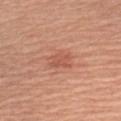Cropped from a whole-body photographic skin survey; the tile spans about 15 mm. The lesion-visualizer software estimated a border-irregularity index near 3/10, a color-variation rating of about 2.5/10, and a peripheral color-asymmetry measure near 1. The software also gave an automated nevus-likeness rating near 15 out of 100. Captured under white-light illumination. The lesion is located on the left upper arm. A female patient in their mid-50s.